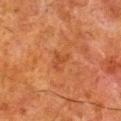The lesion is on the right lower leg.
The patient is a male about 80 years old.
A close-up tile cropped from a whole-body skin photograph, about 15 mm across.